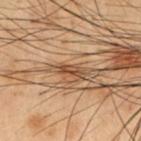Q: Was this lesion biopsied?
A: no biopsy performed (imaged during a skin exam)
Q: Automated lesion metrics?
A: a symmetry-axis asymmetry near 0.3
Q: What kind of image is this?
A: total-body-photography crop, ~15 mm field of view
Q: Patient demographics?
A: male, aged 53–57
Q: How large is the lesion?
A: about 2.5 mm
Q: Illumination type?
A: cross-polarized
Q: What is the anatomic site?
A: the chest